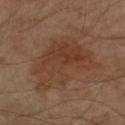The lesion was photographed on a routine skin check and not biopsied; there is no pathology result.
A male patient roughly 70 years of age.
About 8.5 mm across.
Located on the right upper arm.
A 15 mm close-up tile from a total-body photography series done for melanoma screening.
Captured under cross-polarized illumination.